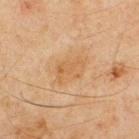Q: Is there a histopathology result?
A: no biopsy performed (imaged during a skin exam)
Q: What is the imaging modality?
A: total-body-photography crop, ~15 mm field of view
Q: What is the anatomic site?
A: the upper back
Q: Automated lesion metrics?
A: an average lesion color of about L≈49 a*≈16 b*≈33 (CIELAB), a lesion–skin lightness drop of about 5, and a lesion-to-skin contrast of about 5 (normalized; higher = more distinct); a border-irregularity index near 3/10, a within-lesion color-variation index near 2/10, and peripheral color asymmetry of about 0.5; a classifier nevus-likeness of about 0/100 and a lesion-detection confidence of about 100/100
Q: Lesion size?
A: ≈4 mm
Q: Who is the patient?
A: male, aged approximately 45
Q: Illumination type?
A: cross-polarized illumination